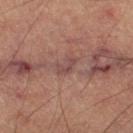Clinical impression:
No biopsy was performed on this lesion — it was imaged during a full skin examination and was not determined to be concerning.
Clinical summary:
A region of skin cropped from a whole-body photographic capture, roughly 15 mm wide. Captured under cross-polarized illumination. A male subject aged approximately 65. The lesion-visualizer software estimated a footprint of about 3.5 mm², a shape eccentricity near 0.8, and two-axis asymmetry of about 0.35. It also reported a mean CIELAB color near L≈46 a*≈20 b*≈21, roughly 7 lightness units darker than nearby skin, and a lesion-to-skin contrast of about 6 (normalized; higher = more distinct). The software also gave a classifier nevus-likeness of about 0/100 and lesion-presence confidence of about 75/100. Located on the right thigh.